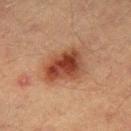Imaged during a routine full-body skin examination; the lesion was not biopsied and no histopathology is available.
The lesion is located on the right thigh.
The lesion-visualizer software estimated an area of roughly 14 mm², a shape eccentricity near 0.7, and a symmetry-axis asymmetry near 0.3. The software also gave a mean CIELAB color near L≈36 a*≈23 b*≈28, about 12 CIELAB-L* units darker than the surrounding skin, and a lesion-to-skin contrast of about 10.5 (normalized; higher = more distinct). It also reported a color-variation rating of about 6/10 and radial color variation of about 2. It also reported a nevus-likeness score of about 100/100 and a detector confidence of about 100 out of 100 that the crop contains a lesion.
Captured under cross-polarized illumination.
About 5 mm across.
A 15 mm close-up tile from a total-body photography series done for melanoma screening.
A male patient, aged around 40.